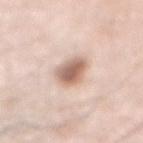notes — total-body-photography surveillance lesion; no biopsy | automated lesion analysis — a shape eccentricity near 0.65 and a symmetry-axis asymmetry near 0.2 | body site — the abdomen | tile lighting — white-light illumination | image — ~15 mm tile from a whole-body skin photo | lesion diameter — ~3.5 mm (longest diameter) | patient — male, in their 80s.